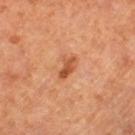Part of a total-body skin-imaging series; this lesion was reviewed on a skin check and was not flagged for biopsy. A female patient, aged 63–67. The lesion is located on the leg. A 15 mm close-up extracted from a 3D total-body photography capture. Captured under cross-polarized illumination. Automated image analysis of the tile measured an area of roughly 4 mm², a shape eccentricity near 0.85, and a shape-asymmetry score of about 0.25 (0 = symmetric). It also reported an average lesion color of about L≈52 a*≈28 b*≈39 (CIELAB), about 11 CIELAB-L* units darker than the surrounding skin, and a normalized border contrast of about 8.